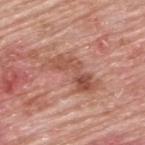Assessment:
The lesion was photographed on a routine skin check and not biopsied; there is no pathology result.
Image and clinical context:
The subject is a male aged approximately 80. A 15 mm crop from a total-body photograph taken for skin-cancer surveillance. This is a white-light tile. Located on the back. The total-body-photography lesion software estimated an area of roughly 12 mm² and an eccentricity of roughly 0.9. The analysis additionally found an average lesion color of about L≈53 a*≈25 b*≈29 (CIELAB), a lesion–skin lightness drop of about 10, and a lesion-to-skin contrast of about 7 (normalized; higher = more distinct). The software also gave a lesion-detection confidence of about 100/100. The lesion's longest dimension is about 6.5 mm.